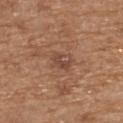| field | value |
|---|---|
| notes | total-body-photography surveillance lesion; no biopsy |
| imaging modality | total-body-photography crop, ~15 mm field of view |
| site | the upper back |
| subject | male, about 70 years old |